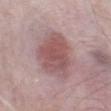Q: What lighting was used for the tile?
A: white-light illumination
Q: Lesion location?
A: the abdomen
Q: What is the imaging modality?
A: 15 mm crop, total-body photography
Q: What did automated image analysis measure?
A: an area of roughly 22 mm², an eccentricity of roughly 0.65, and a shape-asymmetry score of about 0.2 (0 = symmetric)
Q: What are the patient's age and sex?
A: male, in their 60s
Q: Lesion size?
A: about 6.5 mm